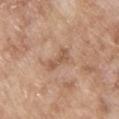notes — total-body-photography surveillance lesion; no biopsy
site — the upper back
imaging modality — total-body-photography crop, ~15 mm field of view
patient — male, in their mid- to late 60s